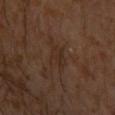<tbp_lesion>
  <biopsy_status>not biopsied; imaged during a skin examination</biopsy_status>
  <lesion_size>
    <long_diameter_mm_approx>2.5</long_diameter_mm_approx>
  </lesion_size>
  <patient>
    <sex>male</sex>
    <age_approx>30</age_approx>
  </patient>
  <site>chest</site>
  <lighting>cross-polarized</lighting>
  <image>
    <source>total-body photography crop</source>
    <field_of_view_mm>15</field_of_view_mm>
  </image>
</tbp_lesion>